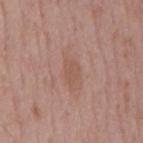Clinical summary:
Located on the mid back. A male subject, about 55 years old. Cropped from a whole-body photographic skin survey; the tile spans about 15 mm.